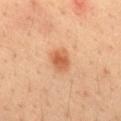biopsy status: total-body-photography surveillance lesion; no biopsy | tile lighting: cross-polarized illumination | lesion size: about 3 mm | automated metrics: a mean CIELAB color near L≈55 a*≈25 b*≈36 and a lesion-to-skin contrast of about 7.5 (normalized; higher = more distinct) | imaging modality: ~15 mm tile from a whole-body skin photo | subject: male, aged 33 to 37 | body site: the back.